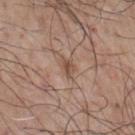The lesion was photographed on a routine skin check and not biopsied; there is no pathology result. This is a white-light tile. An algorithmic analysis of the crop reported two-axis asymmetry of about 0.3. The analysis additionally found about 8 CIELAB-L* units darker than the surrounding skin and a normalized border contrast of about 6. The analysis additionally found a border-irregularity index near 3/10 and a peripheral color-asymmetry measure near 1. The lesion is located on the front of the torso. A region of skin cropped from a whole-body photographic capture, roughly 15 mm wide. The patient is a male in their mid- to late 70s. Longest diameter approximately 2.5 mm.